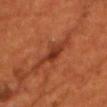No biopsy was performed on this lesion — it was imaged during a full skin examination and was not determined to be concerning. The patient is a male roughly 55 years of age. Automated image analysis of the tile measured an average lesion color of about L≈33 a*≈28 b*≈32 (CIELAB), a lesion–skin lightness drop of about 9, and a normalized lesion–skin contrast near 8.5. The software also gave border irregularity of about 5 on a 0–10 scale, a within-lesion color-variation index near 3.5/10, and radial color variation of about 1. A 15 mm close-up extracted from a 3D total-body photography capture. The lesion's longest dimension is about 4.5 mm. The lesion is on the chest. The tile uses cross-polarized illumination.